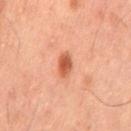Case summary:
* biopsy status — no biopsy performed (imaged during a skin exam)
* subject — male, aged 58 to 62
* site — the mid back
* imaging modality — 15 mm crop, total-body photography